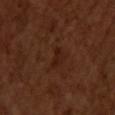<case>
  <biopsy_status>not biopsied; imaged during a skin examination</biopsy_status>
  <patient>
    <sex>male</sex>
    <age_approx>50</age_approx>
  </patient>
  <image>
    <source>total-body photography crop</source>
    <field_of_view_mm>15</field_of_view_mm>
  </image>
  <automated_metrics>
    <area_mm2_approx>3.5</area_mm2_approx>
    <eccentricity>0.85</eccentricity>
    <shape_asymmetry>0.35</shape_asymmetry>
    <cielab_L>20</cielab_L>
    <cielab_a>20</cielab_a>
    <cielab_b>25</cielab_b>
    <vs_skin_contrast_norm>6.0</vs_skin_contrast_norm>
    <nevus_likeness_0_100>5</nevus_likeness_0_100>
    <lesion_detection_confidence_0_100>100</lesion_detection_confidence_0_100>
  </automated_metrics>
  <site>chest</site>
  <lesion_size>
    <long_diameter_mm_approx>2.5</long_diameter_mm_approx>
  </lesion_size>
</case>